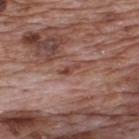notes — total-body-photography surveillance lesion; no biopsy
subject — male, aged 68–72
size — about 2.5 mm
automated metrics — an area of roughly 2.5 mm², a shape eccentricity near 0.9, and a shape-asymmetry score of about 0.4 (0 = symmetric)
site — the upper back
tile lighting — white-light illumination
imaging modality — ~15 mm tile from a whole-body skin photo